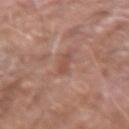{"biopsy_status": "not biopsied; imaged during a skin examination", "patient": {"sex": "male", "age_approx": 75}, "image": {"source": "total-body photography crop", "field_of_view_mm": 15}, "site": "right forearm"}